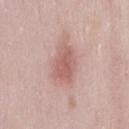  biopsy_status: not biopsied; imaged during a skin examination
  lighting: white-light
  site: right thigh
  automated_metrics:
    area_mm2_approx: 9.5
    eccentricity: 0.7
    shape_asymmetry: 0.25
    vs_skin_darker_L: 10.0
    vs_skin_contrast_norm: 6.5
    border_irregularity_0_10: 2.5
    color_variation_0_10: 2.0
    peripheral_color_asymmetry: 1.0
  patient:
    sex: female
    age_approx: 30
  image:
    source: total-body photography crop
    field_of_view_mm: 15
  lesion_size:
    long_diameter_mm_approx: 4.0